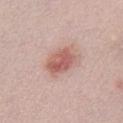A roughly 15 mm field-of-view crop from a total-body skin photograph.
A male subject, roughly 30 years of age.
The tile uses white-light illumination.
About 4 mm across.